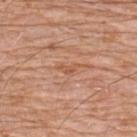{"biopsy_status": "not biopsied; imaged during a skin examination", "patient": {"sex": "male", "age_approx": 80}, "lighting": "white-light", "site": "upper back", "lesion_size": {"long_diameter_mm_approx": 2.5}, "image": {"source": "total-body photography crop", "field_of_view_mm": 15}}